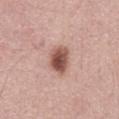Notes:
• follow-up · no biopsy performed (imaged during a skin exam)
• site · the abdomen
• acquisition · total-body-photography crop, ~15 mm field of view
• diameter · ≈3.5 mm
• illumination · white-light illumination
• subject · male, roughly 60 years of age